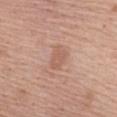illumination: white-light | body site: the front of the torso | subject: female, aged 53–57 | acquisition: ~15 mm tile from a whole-body skin photo | lesion size: ≈3 mm | automated lesion analysis: a mean CIELAB color near L≈58 a*≈21 b*≈29; border irregularity of about 2.5 on a 0–10 scale and a color-variation rating of about 1.5/10; an automated nevus-likeness rating near 0 out of 100 and a detector confidence of about 100 out of 100 that the crop contains a lesion.